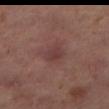Assessment:
Imaged during a routine full-body skin examination; the lesion was not biopsied and no histopathology is available.
Image and clinical context:
A female subject, in their mid-50s. Cropped from a total-body skin-imaging series; the visible field is about 15 mm. Located on the right thigh.